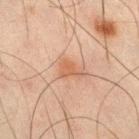Q: Is there a histopathology result?
A: total-body-photography surveillance lesion; no biopsy
Q: Lesion size?
A: ≈2.5 mm
Q: What kind of image is this?
A: total-body-photography crop, ~15 mm field of view
Q: What lighting was used for the tile?
A: cross-polarized illumination
Q: Lesion location?
A: the right thigh
Q: What are the patient's age and sex?
A: male, aged 43 to 47